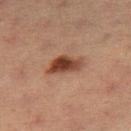Impression:
Part of a total-body skin-imaging series; this lesion was reviewed on a skin check and was not flagged for biopsy.
Image and clinical context:
The tile uses cross-polarized illumination. A roughly 15 mm field-of-view crop from a total-body skin photograph. Located on the right thigh. A female subject aged 53 to 57.